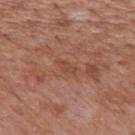No biopsy was performed on this lesion — it was imaged during a full skin examination and was not determined to be concerning. A male patient, in their 70s. A lesion tile, about 15 mm wide, cut from a 3D total-body photograph. On the mid back.